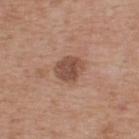– workup: imaged on a skin check; not biopsied
– lighting: white-light illumination
– patient: male, aged 63 to 67
– anatomic site: the back
– image source: ~15 mm crop, total-body skin-cancer survey